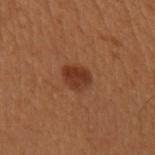Clinical impression:
Captured during whole-body skin photography for melanoma surveillance; the lesion was not biopsied.
Clinical summary:
The lesion's longest dimension is about 3 mm. The patient is a female in their 50s. The tile uses cross-polarized illumination. The lesion is located on the left upper arm. A roughly 15 mm field-of-view crop from a total-body skin photograph.